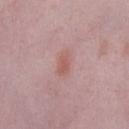biopsy status — catalogued during a skin exam; not biopsied | image-analysis metrics — an average lesion color of about L≈56 a*≈23 b*≈24 (CIELAB), roughly 7 lightness units darker than nearby skin, and a normalized border contrast of about 6; border irregularity of about 2 on a 0–10 scale and internal color variation of about 1.5 on a 0–10 scale; an automated nevus-likeness rating near 45 out of 100 and lesion-presence confidence of about 100/100 | site — the right lower leg | subject — female, in their 40s | image — 15 mm crop, total-body photography | size — ~2.5 mm (longest diameter) | lighting — white-light illumination.